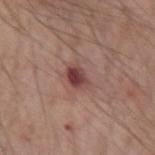Assessment:
Part of a total-body skin-imaging series; this lesion was reviewed on a skin check and was not flagged for biopsy.
Image and clinical context:
The total-body-photography lesion software estimated a footprint of about 4.5 mm², an eccentricity of roughly 0.7, and two-axis asymmetry of about 0.2. The analysis additionally found an average lesion color of about L≈42 a*≈24 b*≈22 (CIELAB), a lesion–skin lightness drop of about 13, and a normalized border contrast of about 10. The analysis additionally found a border-irregularity rating of about 2/10, a within-lesion color-variation index near 5.5/10, and radial color variation of about 2. From the arm. Cropped from a whole-body photographic skin survey; the tile spans about 15 mm. The patient is a male roughly 60 years of age.